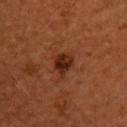Imaged during a routine full-body skin examination; the lesion was not biopsied and no histopathology is available.